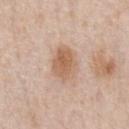| field | value |
|---|---|
| imaging modality | total-body-photography crop, ~15 mm field of view |
| anatomic site | the chest |
| lesion diameter | ~4.5 mm (longest diameter) |
| subject | male, aged 58–62 |